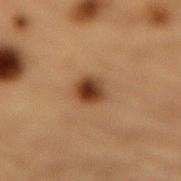<record>
  <biopsy_status>not biopsied; imaged during a skin examination</biopsy_status>
  <automated_metrics>
    <cielab_L>35</cielab_L>
    <cielab_a>19</cielab_a>
    <cielab_b>30</cielab_b>
    <vs_skin_darker_L>13.0</vs_skin_darker_L>
    <vs_skin_contrast_norm>11.5</vs_skin_contrast_norm>
    <border_irregularity_0_10>1.5</border_irregularity_0_10>
    <color_variation_0_10>5.5</color_variation_0_10>
    <peripheral_color_asymmetry>1.5</peripheral_color_asymmetry>
    <lesion_detection_confidence_0_100>100</lesion_detection_confidence_0_100>
  </automated_metrics>
  <lesion_size>
    <long_diameter_mm_approx>2.5</long_diameter_mm_approx>
  </lesion_size>
  <image>
    <source>total-body photography crop</source>
    <field_of_view_mm>15</field_of_view_mm>
  </image>
  <site>lower back</site>
  <patient>
    <sex>male</sex>
    <age_approx>85+</age_approx>
  </patient>
  <lighting>cross-polarized</lighting>
</record>